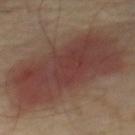<record>
  <biopsy_status>not biopsied; imaged during a skin examination</biopsy_status>
  <lighting>cross-polarized</lighting>
  <patient>
    <sex>male</sex>
    <age_approx>70</age_approx>
  </patient>
  <automated_metrics>
    <area_mm2_approx>60.0</area_mm2_approx>
    <eccentricity>0.85</eccentricity>
    <cielab_L>38</cielab_L>
    <cielab_a>20</cielab_a>
    <cielab_b>22</cielab_b>
    <vs_skin_darker_L>10.0</vs_skin_darker_L>
    <vs_skin_contrast_norm>8.5</vs_skin_contrast_norm>
    <border_irregularity_0_10>3.0</border_irregularity_0_10>
    <color_variation_0_10>5.0</color_variation_0_10>
    <peripheral_color_asymmetry>2.0</peripheral_color_asymmetry>
  </automated_metrics>
  <site>chest</site>
  <lesion_size>
    <long_diameter_mm_approx>12.0</long_diameter_mm_approx>
  </lesion_size>
  <image>
    <source>total-body photography crop</source>
    <field_of_view_mm>15</field_of_view_mm>
  </image>
</record>